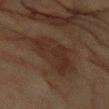subject — female, about 80 years old | lesion diameter — ≈8 mm | location — the arm | lighting — cross-polarized illumination | acquisition — total-body-photography crop, ~15 mm field of view | automated lesion analysis — a mean CIELAB color near L≈24 a*≈14 b*≈22, a lesion–skin lightness drop of about 6, and a lesion-to-skin contrast of about 7.5 (normalized; higher = more distinct).